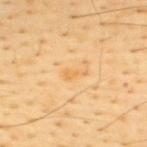acquisition: 15 mm crop, total-body photography
lesion diameter: ~2.5 mm (longest diameter)
illumination: cross-polarized
automated lesion analysis: a lesion area of about 2.5 mm² and two-axis asymmetry of about 0.5; a border-irregularity rating of about 5/10, internal color variation of about 0 on a 0–10 scale, and peripheral color asymmetry of about 0
anatomic site: the upper back
patient: male, about 45 years old